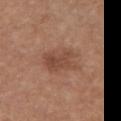| feature | finding |
|---|---|
| workup | catalogued during a skin exam; not biopsied |
| image-analysis metrics | a footprint of about 11 mm², a shape eccentricity near 0.7, and a shape-asymmetry score of about 0.3 (0 = symmetric); an average lesion color of about L≈47 a*≈21 b*≈29 (CIELAB) and a lesion–skin lightness drop of about 8 |
| size | about 4.5 mm |
| tile lighting | white-light illumination |
| acquisition | 15 mm crop, total-body photography |
| location | the chest |
| subject | male, in their mid-60s |